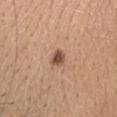Findings:
* workup: total-body-photography surveillance lesion; no biopsy
* patient: female, aged 38 to 42
* site: the head or neck
* imaging modality: total-body-photography crop, ~15 mm field of view